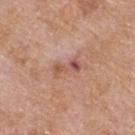Clinical impression:
Imaged during a routine full-body skin examination; the lesion was not biopsied and no histopathology is available.
Background:
A female patient aged around 75. Located on the upper back. This is a white-light tile. Automated tile analysis of the lesion measured about 10 CIELAB-L* units darker than the surrounding skin and a normalized border contrast of about 6.5. The software also gave a nevus-likeness score of about 0/100 and a detector confidence of about 100 out of 100 that the crop contains a lesion. A region of skin cropped from a whole-body photographic capture, roughly 15 mm wide.